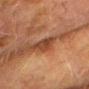illumination: cross-polarized
automated metrics: an eccentricity of roughly 0.4 and a shape-asymmetry score of about 0.35 (0 = symmetric); a normalized border contrast of about 7; a color-variation rating of about 3/10 and a peripheral color-asymmetry measure near 1; an automated nevus-likeness rating near 0 out of 100 and a detector confidence of about 50 out of 100 that the crop contains a lesion
body site: the chest
patient: male, about 75 years old
image: ~15 mm crop, total-body skin-cancer survey
diameter: ~3 mm (longest diameter)